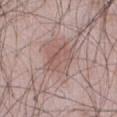Part of a total-body skin-imaging series; this lesion was reviewed on a skin check and was not flagged for biopsy. A male patient, in their 50s. The lesion is on the abdomen. A region of skin cropped from a whole-body photographic capture, roughly 15 mm wide. The lesion-visualizer software estimated an area of roughly 11 mm² and an outline eccentricity of about 0.5 (0 = round, 1 = elongated). It also reported a mean CIELAB color near L≈55 a*≈19 b*≈22, about 7 CIELAB-L* units darker than the surrounding skin, and a normalized lesion–skin contrast near 5. It also reported border irregularity of about 2 on a 0–10 scale, internal color variation of about 4 on a 0–10 scale, and peripheral color asymmetry of about 1.5. The software also gave lesion-presence confidence of about 90/100. The lesion's longest dimension is about 4 mm. This is a white-light tile.